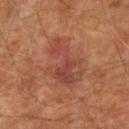follow-up — catalogued during a skin exam; not biopsied | body site — the right upper arm | TBP lesion metrics — a footprint of about 17 mm², an eccentricity of roughly 0.85, and a symmetry-axis asymmetry near 0.35; an average lesion color of about L≈44 a*≈26 b*≈29 (CIELAB) and about 7 CIELAB-L* units darker than the surrounding skin; a border-irregularity index near 6/10, internal color variation of about 5 on a 0–10 scale, and a peripheral color-asymmetry measure near 1.5; a nevus-likeness score of about 0/100 and lesion-presence confidence of about 100/100 | acquisition — total-body-photography crop, ~15 mm field of view | lighting — cross-polarized illumination | patient — male, aged approximately 65 | lesion diameter — ≈6.5 mm.